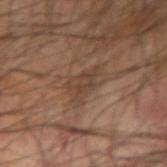biopsy status: catalogued during a skin exam; not biopsied
illumination: cross-polarized
subject: male, in their mid- to late 60s
lesion size: ~4.5 mm (longest diameter)
imaging modality: 15 mm crop, total-body photography
location: the arm